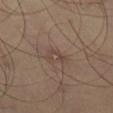follow-up = no biopsy performed (imaged during a skin exam)
site = the leg
subject = male, roughly 45 years of age
diameter = ~2.5 mm (longest diameter)
automated metrics = roughly 6 lightness units darker than nearby skin and a normalized lesion–skin contrast near 5
acquisition = ~15 mm crop, total-body skin-cancer survey
lighting = cross-polarized